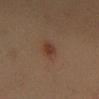<record>
  <biopsy_status>not biopsied; imaged during a skin examination</biopsy_status>
  <patient>
    <sex>female</sex>
    <age_approx>60</age_approx>
  </patient>
  <image>
    <source>total-body photography crop</source>
    <field_of_view_mm>15</field_of_view_mm>
  </image>
  <site>back</site>
  <lesion_size>
    <long_diameter_mm_approx>3.0</long_diameter_mm_approx>
  </lesion_size>
  <automated_metrics>
    <border_irregularity_0_10>2.5</border_irregularity_0_10>
    <color_variation_0_10>3.5</color_variation_0_10>
    <peripheral_color_asymmetry>1.5</peripheral_color_asymmetry>
  </automated_metrics>
  <lighting>cross-polarized</lighting>
</record>